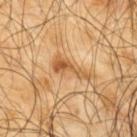follow-up=catalogued during a skin exam; not biopsied | lesion size=≈4.5 mm | image source=15 mm crop, total-body photography | subject=male, in their mid- to late 60s | anatomic site=the upper back.